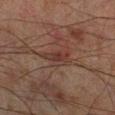<record>
<biopsy_status>not biopsied; imaged during a skin examination</biopsy_status>
<image>
  <source>total-body photography crop</source>
  <field_of_view_mm>15</field_of_view_mm>
</image>
<patient>
  <sex>male</sex>
  <age_approx>70</age_approx>
</patient>
<site>right lower leg</site>
</record>